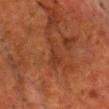patient: male, aged approximately 65; anatomic site: the chest; diameter: ≈5 mm; image: ~15 mm tile from a whole-body skin photo; illumination: cross-polarized.